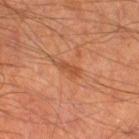This lesion was catalogued during total-body skin photography and was not selected for biopsy.